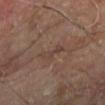Captured during whole-body skin photography for melanoma surveillance; the lesion was not biopsied. A male patient, approximately 70 years of age. The recorded lesion diameter is about 3 mm. This is a cross-polarized tile. Automated tile analysis of the lesion measured a shape eccentricity near 0.85 and two-axis asymmetry of about 0.5. And it measured a mean CIELAB color near L≈39 a*≈16 b*≈22 and a normalized border contrast of about 4.5. The analysis additionally found a border-irregularity rating of about 6/10, a within-lesion color-variation index near 0/10, and radial color variation of about 0. A roughly 15 mm field-of-view crop from a total-body skin photograph. On the left lower leg.